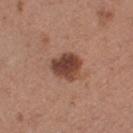Imaged during a routine full-body skin examination; the lesion was not biopsied and no histopathology is available. The recorded lesion diameter is about 4 mm. Automated tile analysis of the lesion measured a footprint of about 9 mm², an eccentricity of roughly 0.65, and two-axis asymmetry of about 0.25. And it measured a border-irregularity rating of about 2.5/10 and peripheral color asymmetry of about 1.5. A 15 mm crop from a total-body photograph taken for skin-cancer surveillance. A female patient, aged 28 to 32. From the right thigh. The tile uses white-light illumination.